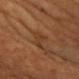Impression:
Part of a total-body skin-imaging series; this lesion was reviewed on a skin check and was not flagged for biopsy.
Acquisition and patient details:
Approximately 4 mm at its widest. This image is a 15 mm lesion crop taken from a total-body photograph. The lesion is on the front of the torso. Imaged with cross-polarized lighting. A male subject, aged approximately 65. The lesion-visualizer software estimated a lesion area of about 5 mm², a shape eccentricity near 0.95, and a symmetry-axis asymmetry near 0.45. And it measured a mean CIELAB color near L≈36 a*≈21 b*≈32, a lesion–skin lightness drop of about 5, and a lesion-to-skin contrast of about 5 (normalized; higher = more distinct). The analysis additionally found a classifier nevus-likeness of about 0/100 and a detector confidence of about 100 out of 100 that the crop contains a lesion.